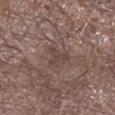Clinical impression: The lesion was tiled from a total-body skin photograph and was not biopsied. Clinical summary: About 3 mm across. Imaged with white-light lighting. From the left forearm. The total-body-photography lesion software estimated an eccentricity of roughly 0.55 and a shape-asymmetry score of about 0.65 (0 = symmetric). It also reported an average lesion color of about L≈43 a*≈16 b*≈21 (CIELAB), roughly 6 lightness units darker than nearby skin, and a normalized lesion–skin contrast near 5.5. And it measured internal color variation of about 2 on a 0–10 scale and peripheral color asymmetry of about 0.5. A male subject, aged around 75. A region of skin cropped from a whole-body photographic capture, roughly 15 mm wide.